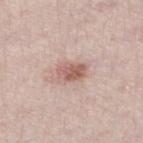workup: no biopsy performed (imaged during a skin exam) | subject: male, aged 58–62 | image: ~15 mm crop, total-body skin-cancer survey | location: the left lower leg | image-analysis metrics: a lesion color around L≈59 a*≈20 b*≈25 in CIELAB; an automated nevus-likeness rating near 80 out of 100 and a detector confidence of about 100 out of 100 that the crop contains a lesion | illumination: white-light | lesion diameter: about 3.5 mm.